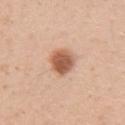Findings:
- biopsy status · imaged on a skin check; not biopsied
- lighting · white-light illumination
- lesion diameter · ≈3.5 mm
- patient · female, aged approximately 25
- acquisition · ~15 mm tile from a whole-body skin photo
- location · the right upper arm